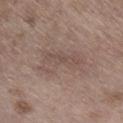biopsy status = total-body-photography surveillance lesion; no biopsy | image source = 15 mm crop, total-body photography | tile lighting = white-light illumination | TBP lesion metrics = a lesion area of about 14 mm², an eccentricity of roughly 0.9, and a symmetry-axis asymmetry near 0.35; a lesion color around L≈50 a*≈15 b*≈22 in CIELAB and roughly 7 lightness units darker than nearby skin; border irregularity of about 5 on a 0–10 scale, a color-variation rating of about 3/10, and radial color variation of about 1; a nevus-likeness score of about 0/100 and a detector confidence of about 100 out of 100 that the crop contains a lesion | subject = male, about 70 years old | anatomic site = the right lower leg.